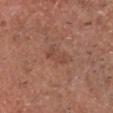Recorded during total-body skin imaging; not selected for excision or biopsy. Captured under white-light illumination. The lesion's longest dimension is about 3 mm. A 15 mm crop from a total-body photograph taken for skin-cancer surveillance. A male subject aged approximately 65. The lesion is located on the head or neck.